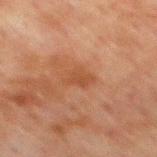Captured during whole-body skin photography for melanoma surveillance; the lesion was not biopsied. On the back. A region of skin cropped from a whole-body photographic capture, roughly 15 mm wide. The subject is a male in their 60s.